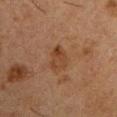Q: Is there a histopathology result?
A: no biopsy performed (imaged during a skin exam)
Q: Lesion location?
A: the left upper arm
Q: Automated lesion metrics?
A: an automated nevus-likeness rating near 0 out of 100 and a lesion-detection confidence of about 100/100
Q: Illumination type?
A: cross-polarized illumination
Q: What are the patient's age and sex?
A: male, in their 50s
Q: How was this image acquired?
A: ~15 mm crop, total-body skin-cancer survey